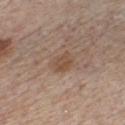  biopsy_status: not biopsied; imaged during a skin examination
  patient:
    sex: male
    age_approx: 60
  image:
    source: total-body photography crop
    field_of_view_mm: 15
  lesion_size:
    long_diameter_mm_approx: 3.0
  lighting: white-light
  site: chest